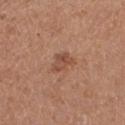notes: no biopsy performed (imaged during a skin exam)
TBP lesion metrics: a lesion color around L≈49 a*≈22 b*≈29 in CIELAB, a lesion–skin lightness drop of about 9, and a lesion-to-skin contrast of about 6.5 (normalized; higher = more distinct); a within-lesion color-variation index near 4.5/10 and peripheral color asymmetry of about 2; a lesion-detection confidence of about 100/100
anatomic site: the left thigh
lesion diameter: ~3 mm (longest diameter)
image source: 15 mm crop, total-body photography
patient: female, approximately 40 years of age